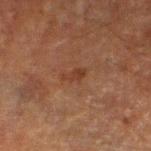Findings:
- workup: no biopsy performed (imaged during a skin exam)
- patient: male, about 60 years old
- diameter: ≈2.5 mm
- automated lesion analysis: an automated nevus-likeness rating near 0 out of 100 and lesion-presence confidence of about 100/100
- anatomic site: the left lower leg
- lighting: cross-polarized illumination
- image source: 15 mm crop, total-body photography